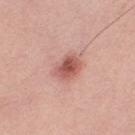Impression: The lesion was photographed on a routine skin check and not biopsied; there is no pathology result. Clinical summary: Automated tile analysis of the lesion measured a lesion area of about 8 mm², a shape eccentricity near 0.65, and two-axis asymmetry of about 0.2. And it measured a lesion color around L≈57 a*≈26 b*≈26 in CIELAB, a lesion–skin lightness drop of about 12, and a normalized border contrast of about 8. It also reported a border-irregularity index near 2/10 and radial color variation of about 1.5. Imaged with white-light lighting. The lesion's longest dimension is about 3.5 mm. A female patient aged around 45. A 15 mm close-up extracted from a 3D total-body photography capture. The lesion is located on the right thigh.